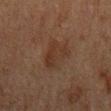Notes:
• patient · male, in their mid- to late 70s
• body site · the mid back
• size · ≈5 mm
• image source · ~15 mm crop, total-body skin-cancer survey
• automated lesion analysis · a shape eccentricity near 0.75 and two-axis asymmetry of about 0.25; a lesion color around L≈27 a*≈14 b*≈22 in CIELAB, roughly 5 lightness units darker than nearby skin, and a lesion-to-skin contrast of about 5.5 (normalized; higher = more distinct); a border-irregularity rating of about 3/10, a color-variation rating of about 3/10, and radial color variation of about 1; a nevus-likeness score of about 5/100
• tile lighting · cross-polarized illumination